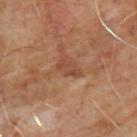{
  "biopsy_status": "not biopsied; imaged during a skin examination",
  "site": "upper back",
  "lesion_size": {
    "long_diameter_mm_approx": 3.0
  },
  "patient": {
    "sex": "male",
    "age_approx": 65
  },
  "image": {
    "source": "total-body photography crop",
    "field_of_view_mm": 15
  }
}